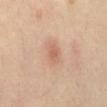Assessment: Recorded during total-body skin imaging; not selected for excision or biopsy. Image and clinical context: From the front of the torso. This is a cross-polarized tile. A female subject, approximately 40 years of age. A 15 mm close-up tile from a total-body photography series done for melanoma screening. Longest diameter approximately 2 mm.